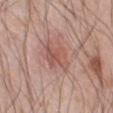Findings:
* workup · catalogued during a skin exam; not biopsied
* patient · male, roughly 60 years of age
* image · ~15 mm crop, total-body skin-cancer survey
* lighting · white-light
* body site · the abdomen